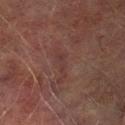workup=no biopsy performed (imaged during a skin exam); size=≈3.5 mm; patient=male, aged 73–77; anatomic site=the left lower leg; tile lighting=cross-polarized illumination; acquisition=15 mm crop, total-body photography.